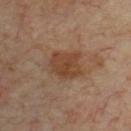biopsy status: total-body-photography surveillance lesion; no biopsy | illumination: cross-polarized illumination | subject: male, in their 70s | imaging modality: total-body-photography crop, ~15 mm field of view | image-analysis metrics: a footprint of about 10 mm², an eccentricity of roughly 0.5, and two-axis asymmetry of about 0.3 | lesion size: about 4 mm | site: the chest.